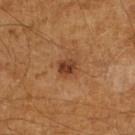Q: Was a biopsy performed?
A: total-body-photography surveillance lesion; no biopsy